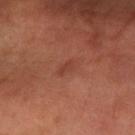The lesion is on the arm.
A male subject, about 55 years old.
The recorded lesion diameter is about 2.5 mm.
Captured under cross-polarized illumination.
A 15 mm close-up extracted from a 3D total-body photography capture.